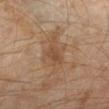Measured at roughly 3 mm in maximum diameter. A region of skin cropped from a whole-body photographic capture, roughly 15 mm wide. The lesion is on the left lower leg. Imaged with cross-polarized lighting. A male subject aged around 60.